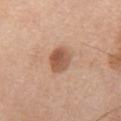Case summary:
– workup: imaged on a skin check; not biopsied
– subject: male, about 75 years old
– image: total-body-photography crop, ~15 mm field of view
– site: the chest